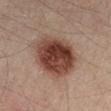Assessment:
Part of a total-body skin-imaging series; this lesion was reviewed on a skin check and was not flagged for biopsy.
Acquisition and patient details:
A 15 mm close-up extracted from a 3D total-body photography capture. A male patient, roughly 40 years of age. From the leg.